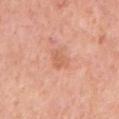notes: catalogued during a skin exam; not biopsied
location: the right upper arm
image source: total-body-photography crop, ~15 mm field of view
diameter: about 3 mm
subject: female, approximately 50 years of age
illumination: white-light
automated lesion analysis: a border-irregularity rating of about 3/10, internal color variation of about 1.5 on a 0–10 scale, and peripheral color asymmetry of about 0.5; a classifier nevus-likeness of about 0/100 and a detector confidence of about 100 out of 100 that the crop contains a lesion